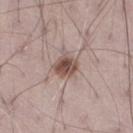A 15 mm close-up extracted from a 3D total-body photography capture. The recorded lesion diameter is about 3 mm. A male patient, about 70 years old. Imaged with white-light lighting. The lesion is on the leg.